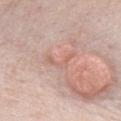Clinical impression: Captured during whole-body skin photography for melanoma surveillance; the lesion was not biopsied. Image and clinical context: Captured under white-light illumination. Measured at roughly 3.5 mm in maximum diameter. A close-up tile cropped from a whole-body skin photograph, about 15 mm across. The patient is a female aged 63–67. An algorithmic analysis of the crop reported a border-irregularity rating of about 8.5/10, internal color variation of about 0 on a 0–10 scale, and peripheral color asymmetry of about 0. It also reported a classifier nevus-likeness of about 0/100 and a lesion-detection confidence of about 50/100. On the chest.